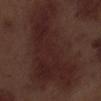biopsy status — no biopsy performed (imaged during a skin exam)